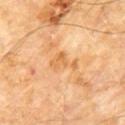Assessment:
The lesion was photographed on a routine skin check and not biopsied; there is no pathology result.
Clinical summary:
Approximately 3.5 mm at its widest. A roughly 15 mm field-of-view crop from a total-body skin photograph. The lesion-visualizer software estimated a classifier nevus-likeness of about 0/100 and a lesion-detection confidence of about 100/100. A male subject, in their 60s. Captured under cross-polarized illumination. From the front of the torso.